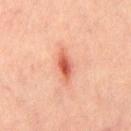follow-up = no biopsy performed (imaged during a skin exam); anatomic site = the front of the torso; patient = male, in their mid-40s; image source = total-body-photography crop, ~15 mm field of view.